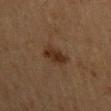This lesion was catalogued during total-body skin photography and was not selected for biopsy. Longest diameter approximately 3 mm. The patient is a male approximately 60 years of age. A 15 mm close-up tile from a total-body photography series done for melanoma screening.